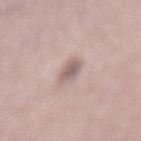Assessment:
The lesion was tiled from a total-body skin photograph and was not biopsied.
Acquisition and patient details:
A female patient, about 65 years old. On the mid back. The recorded lesion diameter is about 3 mm. Imaged with white-light lighting. A 15 mm crop from a total-body photograph taken for skin-cancer surveillance.